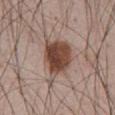Q: Was this lesion biopsied?
A: no biopsy performed (imaged during a skin exam)
Q: What is the anatomic site?
A: the abdomen
Q: Patient demographics?
A: male, approximately 65 years of age
Q: How was this image acquired?
A: ~15 mm crop, total-body skin-cancer survey
Q: Lesion size?
A: ≈5.5 mm
Q: What did automated image analysis measure?
A: a lesion color around L≈45 a*≈18 b*≈25 in CIELAB; a border-irregularity rating of about 2.5/10 and radial color variation of about 1.5; an automated nevus-likeness rating near 100 out of 100 and a lesion-detection confidence of about 100/100
Q: What lighting was used for the tile?
A: white-light illumination